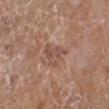  biopsy_status: not biopsied; imaged during a skin examination
  site: left lower leg
  image:
    source: total-body photography crop
    field_of_view_mm: 15
  patient:
    sex: female
    age_approx: 75
  lighting: white-light